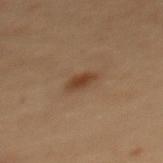Imaged during a routine full-body skin examination; the lesion was not biopsied and no histopathology is available. A 15 mm close-up tile from a total-body photography series done for melanoma screening. A female subject roughly 50 years of age. Located on the upper back.